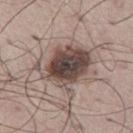Imaged during a routine full-body skin examination; the lesion was not biopsied and no histopathology is available.